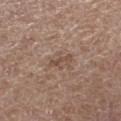<case>
<biopsy_status>not biopsied; imaged during a skin examination</biopsy_status>
<site>left thigh</site>
<patient>
  <sex>female</sex>
  <age_approx>65</age_approx>
</patient>
<image>
  <source>total-body photography crop</source>
  <field_of_view_mm>15</field_of_view_mm>
</image>
</case>